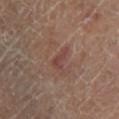Assessment: Imaged during a routine full-body skin examination; the lesion was not biopsied and no histopathology is available. Clinical summary: The subject is female. Longest diameter approximately 2.5 mm. This is a cross-polarized tile. Cropped from a whole-body photographic skin survey; the tile spans about 15 mm. The lesion-visualizer software estimated roughly 7 lightness units darker than nearby skin. The analysis additionally found a detector confidence of about 100 out of 100 that the crop contains a lesion. The lesion is on the left lower leg.